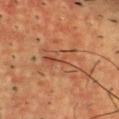Assessment: No biopsy was performed on this lesion — it was imaged during a full skin examination and was not determined to be concerning. Acquisition and patient details: An algorithmic analysis of the crop reported a border-irregularity index near 6.5/10, a within-lesion color-variation index near 6/10, and peripheral color asymmetry of about 2.5. A male patient, approximately 55 years of age. About 5 mm across. A region of skin cropped from a whole-body photographic capture, roughly 15 mm wide. From the upper back.